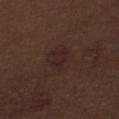This lesion was catalogued during total-body skin photography and was not selected for biopsy.
This is a white-light tile.
A 15 mm close-up tile from a total-body photography series done for melanoma screening.
From the abdomen.
The total-body-photography lesion software estimated a lesion area of about 6.5 mm². And it measured an automated nevus-likeness rating near 55 out of 100 and a detector confidence of about 100 out of 100 that the crop contains a lesion.
The recorded lesion diameter is about 3.5 mm.
A male subject, aged around 70.